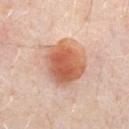Assessment:
The lesion was photographed on a routine skin check and not biopsied; there is no pathology result.